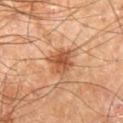This image is a 15 mm lesion crop taken from a total-body photograph.
On the right leg.
The tile uses cross-polarized illumination.
The total-body-photography lesion software estimated a lesion–skin lightness drop of about 11 and a lesion-to-skin contrast of about 7.5 (normalized; higher = more distinct). It also reported a border-irregularity index near 3.5/10 and a color-variation rating of about 4/10.
A male patient, aged around 60.
The lesion's longest dimension is about 4 mm.